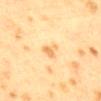<tbp_lesion>
  <image>
    <source>total-body photography crop</source>
    <field_of_view_mm>15</field_of_view_mm>
  </image>
  <site>mid back</site>
  <lesion_size>
    <long_diameter_mm_approx>2.5</long_diameter_mm_approx>
  </lesion_size>
  <patient>
    <sex>female</sex>
    <age_approx>40</age_approx>
  </patient>
  <lighting>cross-polarized</lighting>
</tbp_lesion>